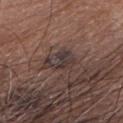No biopsy was performed on this lesion — it was imaged during a full skin examination and was not determined to be concerning.
An algorithmic analysis of the crop reported an area of roughly 7 mm², an outline eccentricity of about 0.8 (0 = round, 1 = elongated), and a shape-asymmetry score of about 0.5 (0 = symmetric). It also reported about 8 CIELAB-L* units darker than the surrounding skin and a lesion-to-skin contrast of about 8.5 (normalized; higher = more distinct). And it measured a border-irregularity index near 6.5/10 and a color-variation rating of about 3.5/10. The analysis additionally found an automated nevus-likeness rating near 0 out of 100.
Cropped from a total-body skin-imaging series; the visible field is about 15 mm.
From the head or neck.
The lesion's longest dimension is about 4 mm.
A female subject aged 73–77.